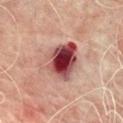The lesion was tiled from a total-body skin photograph and was not biopsied.
On the mid back.
Cropped from a whole-body photographic skin survey; the tile spans about 15 mm.
The patient is a male about 70 years old.